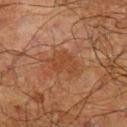follow-up = imaged on a skin check; not biopsied
anatomic site = the left upper arm
image = ~15 mm crop, total-body skin-cancer survey
subject = male, roughly 70 years of age
diameter = ~4 mm (longest diameter)
lighting = cross-polarized